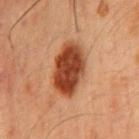biopsy status=imaged on a skin check; not biopsied | TBP lesion metrics=an average lesion color of about L≈33 a*≈22 b*≈29 (CIELAB), roughly 14 lightness units darker than nearby skin, and a normalized lesion–skin contrast near 12.5; border irregularity of about 1.5 on a 0–10 scale, a within-lesion color-variation index near 4/10, and peripheral color asymmetry of about 1.5; a nevus-likeness score of about 100/100 and lesion-presence confidence of about 100/100 | anatomic site=the front of the torso | illumination=cross-polarized illumination | patient=male, in their 50s | image source=total-body-photography crop, ~15 mm field of view | lesion diameter=about 5.5 mm.